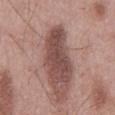workup = catalogued during a skin exam; not biopsied
acquisition = 15 mm crop, total-body photography
image-analysis metrics = a lesion color around L≈47 a*≈21 b*≈22 in CIELAB and about 14 CIELAB-L* units darker than the surrounding skin; border irregularity of about 4.5 on a 0–10 scale, internal color variation of about 3 on a 0–10 scale, and a peripheral color-asymmetry measure near 1; a nevus-likeness score of about 0/100
lesion size = ~8.5 mm (longest diameter)
illumination = white-light
patient = male, in their mid-50s
location = the mid back